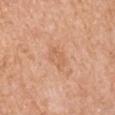notes: no biopsy performed (imaged during a skin exam) | imaging modality: 15 mm crop, total-body photography | anatomic site: the left upper arm | patient: male, about 65 years old | tile lighting: white-light illumination | size: about 3 mm.